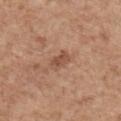Part of a total-body skin-imaging series; this lesion was reviewed on a skin check and was not flagged for biopsy. The lesion's longest dimension is about 3 mm. Cropped from a whole-body photographic skin survey; the tile spans about 15 mm. Imaged with white-light lighting. The lesion is on the right upper arm. The subject is a male aged around 70.